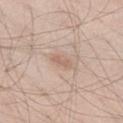automated lesion analysis — border irregularity of about 2.5 on a 0–10 scale, a within-lesion color-variation index near 0/10, and radial color variation of about 0
size — about 2.5 mm
site — the left thigh
image source — 15 mm crop, total-body photography
patient — male, aged around 35
tile lighting — white-light